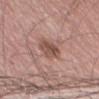Findings:
• notes: catalogued during a skin exam; not biopsied
• size: ≈3.5 mm
• tile lighting: white-light
• acquisition: ~15 mm tile from a whole-body skin photo
• location: the left thigh
• patient: male, aged around 75
• image-analysis metrics: a footprint of about 6.5 mm², an outline eccentricity of about 0.75 (0 = round, 1 = elongated), and a symmetry-axis asymmetry near 0.15